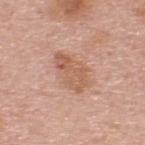Assessment: This lesion was catalogued during total-body skin photography and was not selected for biopsy. Clinical summary: The patient is a male about 65 years old. The tile uses white-light illumination. From the upper back. The recorded lesion diameter is about 5 mm. This image is a 15 mm lesion crop taken from a total-body photograph. Automated image analysis of the tile measured an average lesion color of about L≈59 a*≈22 b*≈31 (CIELAB), roughly 9 lightness units darker than nearby skin, and a normalized lesion–skin contrast near 6.5. The software also gave an automated nevus-likeness rating near 0 out of 100.